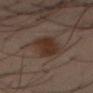  site: abdomen
  patient:
    sex: male
    age_approx: 40
  image:
    source: total-body photography crop
    field_of_view_mm: 15
  automated_metrics:
    area_mm2_approx: 13.0
    shape_asymmetry: 0.2
    border_irregularity_0_10: 3.0
    peripheral_color_asymmetry: 1.0
    nevus_likeness_0_100: 90
    lesion_detection_confidence_0_100: 100
  lesion_size:
    long_diameter_mm_approx: 5.5